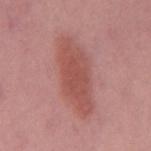{"biopsy_status": "not biopsied; imaged during a skin examination", "lighting": "white-light", "patient": {"sex": "male", "age_approx": 55}, "lesion_size": {"long_diameter_mm_approx": 8.0}, "site": "mid back", "automated_metrics": {"area_mm2_approx": 21.0, "shape_asymmetry": 0.2, "cielab_L": 52, "cielab_a": 27, "cielab_b": 25, "vs_skin_contrast_norm": 7.0, "border_irregularity_0_10": 3.0, "color_variation_0_10": 2.5, "peripheral_color_asymmetry": 1.0, "nevus_likeness_0_100": 70, "lesion_detection_confidence_0_100": 100}, "image": {"source": "total-body photography crop", "field_of_view_mm": 15}}